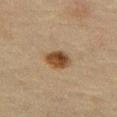Case summary:
* notes: imaged on a skin check; not biopsied
* image source: 15 mm crop, total-body photography
* image-analysis metrics: a lesion area of about 11 mm² and an outline eccentricity of about 0.5 (0 = round, 1 = elongated); about 9 CIELAB-L* units darker than the surrounding skin and a normalized border contrast of about 8; a border-irregularity index near 2/10 and internal color variation of about 7 on a 0–10 scale
* anatomic site: the abdomen
* patient: male, roughly 70 years of age